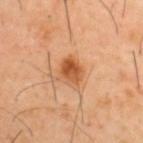Q: Is there a histopathology result?
A: imaged on a skin check; not biopsied
Q: Who is the patient?
A: male, aged around 40
Q: How was this image acquired?
A: 15 mm crop, total-body photography
Q: What is the anatomic site?
A: the upper back